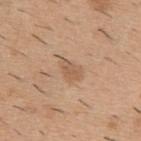Q: Was a biopsy performed?
A: imaged on a skin check; not biopsied
Q: What is the imaging modality?
A: total-body-photography crop, ~15 mm field of view
Q: What is the anatomic site?
A: the upper back
Q: What lighting was used for the tile?
A: white-light illumination
Q: What are the patient's age and sex?
A: male, in their 40s
Q: Lesion size?
A: about 2.5 mm